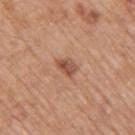follow-up = total-body-photography surveillance lesion; no biopsy
site = the right upper arm
lighting = white-light
patient = male, about 65 years old
diameter = ≈2.5 mm
image source = total-body-photography crop, ~15 mm field of view